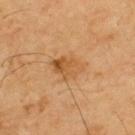biopsy status: catalogued during a skin exam; not biopsied
body site: the upper back
subject: male, aged around 70
imaging modality: ~15 mm tile from a whole-body skin photo
lighting: cross-polarized illumination
automated metrics: an area of roughly 8.5 mm², an eccentricity of roughly 0.75, and a symmetry-axis asymmetry near 0.2; a lesion-to-skin contrast of about 6.5 (normalized; higher = more distinct); a border-irregularity index near 2.5/10, a color-variation rating of about 7/10, and radial color variation of about 3
lesion size: ≈4 mm